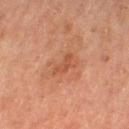Findings:
• tile lighting — cross-polarized illumination
• lesion size — ≈3 mm
• TBP lesion metrics — a nevus-likeness score of about 0/100 and lesion-presence confidence of about 100/100
• subject — female, in their mid-50s
• body site — the leg
• image source — ~15 mm tile from a whole-body skin photo